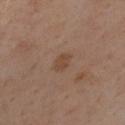| key | value |
|---|---|
| biopsy status | total-body-photography surveillance lesion; no biopsy |
| image source | total-body-photography crop, ~15 mm field of view |
| lesion diameter | ~2.5 mm (longest diameter) |
| site | the chest |
| lighting | cross-polarized illumination |
| patient | male, about 55 years old |